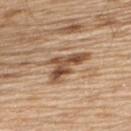Impression:
No biopsy was performed on this lesion — it was imaged during a full skin examination and was not determined to be concerning.
Context:
On the upper back. Longest diameter approximately 5.5 mm. Captured under white-light illumination. Cropped from a whole-body photographic skin survey; the tile spans about 15 mm. The patient is a male aged 68 to 72. The lesion-visualizer software estimated a footprint of about 9 mm².